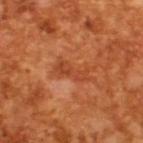| feature | finding |
|---|---|
| follow-up | no biopsy performed (imaged during a skin exam) |
| subject | male, about 65 years old |
| image source | ~15 mm tile from a whole-body skin photo |
| lighting | cross-polarized illumination |